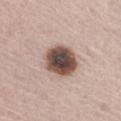workup = imaged on a skin check; not biopsied
automated lesion analysis = a detector confidence of about 100 out of 100 that the crop contains a lesion
lesion diameter = ≈4 mm
lighting = white-light
body site = the abdomen
patient = male, in their mid- to late 60s
image source = ~15 mm crop, total-body skin-cancer survey